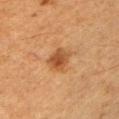| field | value |
|---|---|
| workup | total-body-photography surveillance lesion; no biopsy |
| site | the left upper arm |
| subject | male, about 50 years old |
| lesion diameter | about 3 mm |
| tile lighting | cross-polarized |
| image source | total-body-photography crop, ~15 mm field of view |